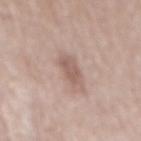Q: Is there a histopathology result?
A: imaged on a skin check; not biopsied
Q: Lesion size?
A: ≈3.5 mm
Q: Lesion location?
A: the mid back
Q: What is the imaging modality?
A: 15 mm crop, total-body photography
Q: How was the tile lit?
A: white-light
Q: What are the patient's age and sex?
A: male, about 80 years old